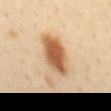| feature | finding |
|---|---|
| patient | female, aged 38–42 |
| image | ~15 mm crop, total-body skin-cancer survey |
| site | the mid back |